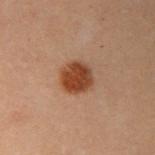* biopsy status — catalogued during a skin exam; not biopsied
* subject — female, aged 28 to 32
* acquisition — ~15 mm crop, total-body skin-cancer survey
* TBP lesion metrics — an area of roughly 9 mm², an outline eccentricity of about 0.45 (0 = round, 1 = elongated), and a symmetry-axis asymmetry near 0.15; a border-irregularity rating of about 1.5/10 and internal color variation of about 3 on a 0–10 scale; an automated nevus-likeness rating near 100 out of 100 and a detector confidence of about 100 out of 100 that the crop contains a lesion
* body site — the left arm
* size — ~3.5 mm (longest diameter)
* lighting — cross-polarized